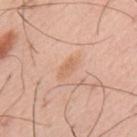Part of a total-body skin-imaging series; this lesion was reviewed on a skin check and was not flagged for biopsy.
Located on the mid back.
A male patient aged 53 to 57.
Imaged with white-light lighting.
Measured at roughly 3 mm in maximum diameter.
The total-body-photography lesion software estimated border irregularity of about 3 on a 0–10 scale, a within-lesion color-variation index near 1.5/10, and radial color variation of about 0.5.
A roughly 15 mm field-of-view crop from a total-body skin photograph.